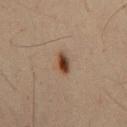Q: Was this lesion biopsied?
A: total-body-photography surveillance lesion; no biopsy
Q: What is the anatomic site?
A: the mid back
Q: What are the patient's age and sex?
A: male, aged 48 to 52
Q: What is the imaging modality?
A: ~15 mm tile from a whole-body skin photo